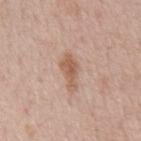No biopsy was performed on this lesion — it was imaged during a full skin examination and was not determined to be concerning. Located on the chest. The tile uses white-light illumination. The lesion-visualizer software estimated an eccentricity of roughly 0.9. The analysis additionally found a classifier nevus-likeness of about 75/100 and a detector confidence of about 100 out of 100 that the crop contains a lesion. A male subject, in their mid-60s. Measured at roughly 4 mm in maximum diameter. A region of skin cropped from a whole-body photographic capture, roughly 15 mm wide.